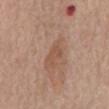Q: Was a biopsy performed?
A: catalogued during a skin exam; not biopsied
Q: Lesion location?
A: the mid back
Q: Lesion size?
A: ≈2.5 mm
Q: How was the tile lit?
A: white-light illumination
Q: Patient demographics?
A: male, aged 63 to 67
Q: How was this image acquired?
A: 15 mm crop, total-body photography